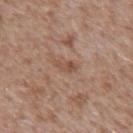Clinical impression:
The lesion was tiled from a total-body skin photograph and was not biopsied.
Background:
The tile uses white-light illumination. From the mid back. Measured at roughly 3 mm in maximum diameter. Cropped from a total-body skin-imaging series; the visible field is about 15 mm. A male subject aged around 65.